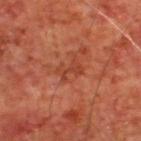The lesion was tiled from a total-body skin photograph and was not biopsied.
An algorithmic analysis of the crop reported an average lesion color of about L≈38 a*≈28 b*≈32 (CIELAB) and roughly 6 lightness units darker than nearby skin. The analysis additionally found an automated nevus-likeness rating near 0 out of 100.
The lesion is on the upper back.
Imaged with cross-polarized lighting.
The lesion's longest dimension is about 3 mm.
A male subject, aged 63–67.
A 15 mm crop from a total-body photograph taken for skin-cancer surveillance.